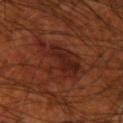{
  "biopsy_status": "not biopsied; imaged during a skin examination",
  "image": {
    "source": "total-body photography crop",
    "field_of_view_mm": 15
  },
  "site": "left forearm",
  "automated_metrics": {
    "vs_skin_darker_L": 6.0,
    "vs_skin_contrast_norm": 7.5,
    "border_irregularity_0_10": 6.0,
    "color_variation_0_10": 4.5,
    "peripheral_color_asymmetry": 1.5
  },
  "lesion_size": {
    "long_diameter_mm_approx": 5.5
  },
  "patient": {
    "sex": "male",
    "age_approx": 70
  },
  "lighting": "cross-polarized"
}